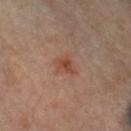Impression: The lesion was photographed on a routine skin check and not biopsied; there is no pathology result. Acquisition and patient details: Located on the left forearm. A roughly 15 mm field-of-view crop from a total-body skin photograph. Approximately 2.5 mm at its widest. The patient is a male aged 63–67.